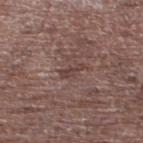| key | value |
|---|---|
| biopsy status | imaged on a skin check; not biopsied |
| diameter | ~3 mm (longest diameter) |
| patient | male, approximately 70 years of age |
| anatomic site | the left lower leg |
| image | ~15 mm tile from a whole-body skin photo |